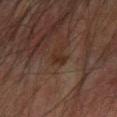The lesion was photographed on a routine skin check and not biopsied; there is no pathology result.
On the left forearm.
A male subject, aged around 75.
The lesion-visualizer software estimated a symmetry-axis asymmetry near 0.35. The software also gave an average lesion color of about L≈24 a*≈15 b*≈22 (CIELAB) and about 5 CIELAB-L* units darker than the surrounding skin. It also reported a border-irregularity rating of about 3.5/10, internal color variation of about 1.5 on a 0–10 scale, and radial color variation of about 0.5.
The tile uses cross-polarized illumination.
About 2.5 mm across.
A lesion tile, about 15 mm wide, cut from a 3D total-body photograph.